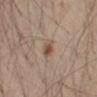This lesion was catalogued during total-body skin photography and was not selected for biopsy.
The lesion is located on the mid back.
Longest diameter approximately 2.5 mm.
Cropped from a total-body skin-imaging series; the visible field is about 15 mm.
This is a white-light tile.
An algorithmic analysis of the crop reported an area of roughly 4 mm², an eccentricity of roughly 0.65, and a symmetry-axis asymmetry near 0.25. And it measured an average lesion color of about L≈51 a*≈15 b*≈27 (CIELAB), about 10 CIELAB-L* units darker than the surrounding skin, and a normalized lesion–skin contrast near 7.5. The software also gave internal color variation of about 3 on a 0–10 scale and peripheral color asymmetry of about 1. And it measured a nevus-likeness score of about 90/100 and lesion-presence confidence of about 100/100.
The subject is a male roughly 50 years of age.